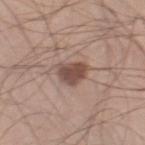Q: Is there a histopathology result?
A: imaged on a skin check; not biopsied
Q: What is the anatomic site?
A: the leg
Q: Who is the patient?
A: male, aged around 30
Q: What kind of image is this?
A: ~15 mm crop, total-body skin-cancer survey
Q: How was the tile lit?
A: white-light illumination
Q: Lesion size?
A: ~3.5 mm (longest diameter)
Q: Automated lesion metrics?
A: border irregularity of about 3 on a 0–10 scale, a color-variation rating of about 2.5/10, and a peripheral color-asymmetry measure near 1